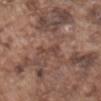  biopsy_status: not biopsied; imaged during a skin examination
  lesion_size:
    long_diameter_mm_approx: 3.0
  image:
    source: total-body photography crop
    field_of_view_mm: 15
  patient:
    sex: male
    age_approx: 75
  site: abdomen